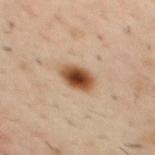Recorded during total-body skin imaging; not selected for excision or biopsy.
The subject is a male aged 38 to 42.
Cropped from a whole-body photographic skin survey; the tile spans about 15 mm.
The lesion is located on the upper back.